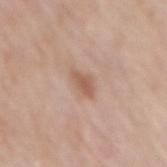The lesion was photographed on a routine skin check and not biopsied; there is no pathology result.
The lesion's longest dimension is about 2.5 mm.
A female subject, aged 73 to 77.
Cropped from a whole-body photographic skin survey; the tile spans about 15 mm.
The lesion is located on the mid back.